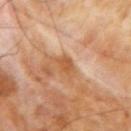This lesion was catalogued during total-body skin photography and was not selected for biopsy.
The lesion's longest dimension is about 3 mm.
A male subject roughly 70 years of age.
This is a cross-polarized tile.
The total-body-photography lesion software estimated a lesion area of about 4.5 mm², an eccentricity of roughly 0.7, and a shape-asymmetry score of about 0.4 (0 = symmetric). It also reported a border-irregularity rating of about 4/10, a color-variation rating of about 2/10, and a peripheral color-asymmetry measure near 0.5. It also reported a nevus-likeness score of about 0/100 and lesion-presence confidence of about 100/100.
A 15 mm close-up extracted from a 3D total-body photography capture.
From the left forearm.